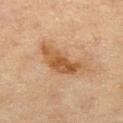biopsy_status: not biopsied; imaged during a skin examination